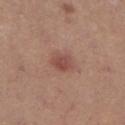Q: Was this lesion biopsied?
A: no biopsy performed (imaged during a skin exam)
Q: What kind of image is this?
A: ~15 mm crop, total-body skin-cancer survey
Q: What are the patient's age and sex?
A: female, in their mid-40s
Q: What lighting was used for the tile?
A: white-light
Q: Lesion size?
A: ≈3 mm
Q: Lesion location?
A: the right lower leg